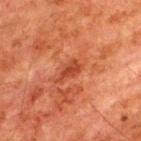<record>
<biopsy_status>not biopsied; imaged during a skin examination</biopsy_status>
<lighting>cross-polarized</lighting>
<lesion_size>
  <long_diameter_mm_approx>3.0</long_diameter_mm_approx>
</lesion_size>
<site>upper back</site>
<image>
  <source>total-body photography crop</source>
  <field_of_view_mm>15</field_of_view_mm>
</image>
<patient>
  <sex>male</sex>
  <age_approx>80</age_approx>
</patient>
</record>